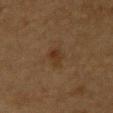The lesion-visualizer software estimated an area of roughly 4.5 mm² and an outline eccentricity of about 0.65 (0 = round, 1 = elongated). It also reported a mean CIELAB color near L≈29 a*≈14 b*≈26, roughly 5 lightness units darker than nearby skin, and a normalized lesion–skin contrast near 6. The analysis additionally found a border-irregularity rating of about 3/10, a color-variation rating of about 2.5/10, and radial color variation of about 1. The software also gave an automated nevus-likeness rating near 35 out of 100 and lesion-presence confidence of about 100/100.
Approximately 3 mm at its widest.
The subject is a male approximately 85 years of age or older.
Cropped from a total-body skin-imaging series; the visible field is about 15 mm.
Imaged with cross-polarized lighting.
On the upper back.